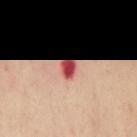Context: Measured at roughly 2 mm in maximum diameter. A male subject, in their mid- to late 40s. The lesion is on the mid back. A close-up tile cropped from a whole-body skin photograph, about 15 mm across.